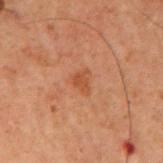Captured during whole-body skin photography for melanoma surveillance; the lesion was not biopsied. The lesion-visualizer software estimated a footprint of about 3 mm², an outline eccentricity of about 0.75 (0 = round, 1 = elongated), and a shape-asymmetry score of about 0.4 (0 = symmetric). It also reported a lesion color around L≈38 a*≈22 b*≈30 in CIELAB and a lesion–skin lightness drop of about 6. And it measured a nevus-likeness score of about 10/100 and a lesion-detection confidence of about 100/100. Captured under cross-polarized illumination. Approximately 2.5 mm at its widest. Cropped from a whole-body photographic skin survey; the tile spans about 15 mm. The subject is a male aged around 60. The lesion is on the right upper arm.